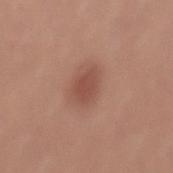Case summary:
* workup · total-body-photography surveillance lesion; no biopsy
* subject · male, roughly 30 years of age
* image source · total-body-photography crop, ~15 mm field of view
* anatomic site · the mid back
* diameter · ≈3 mm